From the right upper arm · the subject is a male about 60 years old · a 15 mm close-up tile from a total-body photography series done for melanoma screening · the recorded lesion diameter is about 5.5 mm.
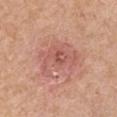Histopathological examination showed an invasive squamous cell carcinoma — a malignant skin lesion.